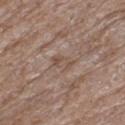The lesion was photographed on a routine skin check and not biopsied; there is no pathology result.
The lesion-visualizer software estimated an area of roughly 5 mm², an outline eccentricity of about 0.75 (0 = round, 1 = elongated), and a shape-asymmetry score of about 0.25 (0 = symmetric). It also reported an average lesion color of about L≈51 a*≈15 b*≈25 (CIELAB) and a normalized lesion–skin contrast near 5.
Captured under white-light illumination.
A 15 mm crop from a total-body photograph taken for skin-cancer surveillance.
The patient is a male roughly 80 years of age.
The lesion's longest dimension is about 3 mm.
The lesion is on the left thigh.